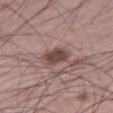Clinical impression: Recorded during total-body skin imaging; not selected for excision or biopsy. Clinical summary: A male patient, in their 60s. A region of skin cropped from a whole-body photographic capture, roughly 15 mm wide. Measured at roughly 3 mm in maximum diameter. From the right thigh. Imaged with white-light lighting.